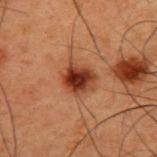notes: catalogued during a skin exam; not biopsied
acquisition: 15 mm crop, total-body photography
diameter: about 3.5 mm
image-analysis metrics: an average lesion color of about L≈29 a*≈21 b*≈26 (CIELAB) and a normalized border contrast of about 11; a border-irregularity rating of about 2/10, internal color variation of about 6.5 on a 0–10 scale, and radial color variation of about 2; a nevus-likeness score of about 100/100 and a detector confidence of about 100 out of 100 that the crop contains a lesion
patient: male, approximately 50 years of age
body site: the back
illumination: cross-polarized